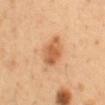– imaging modality: total-body-photography crop, ~15 mm field of view
– location: the abdomen
– patient: male, aged 48 to 52